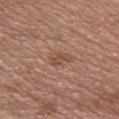follow-up = total-body-photography surveillance lesion; no biopsy
tile lighting = white-light
patient = female, aged around 40
image source = total-body-photography crop, ~15 mm field of view
anatomic site = the chest
diameter = about 3 mm